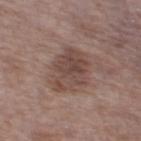| field | value |
|---|---|
| image | ~15 mm tile from a whole-body skin photo |
| patient | male, roughly 75 years of age |
| lesion size | ~6 mm (longest diameter) |
| body site | the left thigh |
| automated metrics | an area of roughly 17 mm² and an eccentricity of roughly 0.75; a mean CIELAB color near L≈45 a*≈17 b*≈22, about 9 CIELAB-L* units darker than the surrounding skin, and a lesion-to-skin contrast of about 7 (normalized; higher = more distinct); a border-irregularity rating of about 2.5/10, a within-lesion color-variation index near 4.5/10, and radial color variation of about 1.5; lesion-presence confidence of about 100/100 |